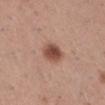The lesion was photographed on a routine skin check and not biopsied; there is no pathology result.
The lesion is on the right lower leg.
A 15 mm crop from a total-body photograph taken for skin-cancer surveillance.
A female patient, approximately 30 years of age.